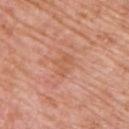No biopsy was performed on this lesion — it was imaged during a full skin examination and was not determined to be concerning. A male subject in their mid- to late 70s. A roughly 15 mm field-of-view crop from a total-body skin photograph. An algorithmic analysis of the crop reported a lesion-to-skin contrast of about 5 (normalized; higher = more distinct). On the upper back. Captured under white-light illumination.